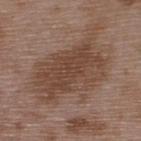Assessment: No biopsy was performed on this lesion — it was imaged during a full skin examination and was not determined to be concerning. Background: An algorithmic analysis of the crop reported a lesion area of about 38 mm², an outline eccentricity of about 0.8 (0 = round, 1 = elongated), and a symmetry-axis asymmetry near 0.25. The analysis additionally found an average lesion color of about L≈44 a*≈17 b*≈26 (CIELAB), a lesion–skin lightness drop of about 9, and a normalized border contrast of about 7. The software also gave a border-irregularity index near 5/10, a color-variation rating of about 3.5/10, and a peripheral color-asymmetry measure near 1.5. The lesion's longest dimension is about 9.5 mm. This image is a 15 mm lesion crop taken from a total-body photograph. Located on the back. The patient is a male aged 48–52. Captured under white-light illumination.